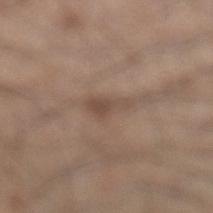biopsy status: imaged on a skin check; not biopsied | site: the left lower leg | lighting: white-light | patient: male, aged approximately 35 | TBP lesion metrics: a lesion color around L≈48 a*≈15 b*≈25 in CIELAB; a border-irregularity rating of about 4.5/10, a color-variation rating of about 1.5/10, and radial color variation of about 0.5; a classifier nevus-likeness of about 0/100 and a detector confidence of about 95 out of 100 that the crop contains a lesion | size: ~3.5 mm (longest diameter) | imaging modality: 15 mm crop, total-body photography.